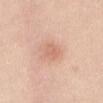Assessment: Part of a total-body skin-imaging series; this lesion was reviewed on a skin check and was not flagged for biopsy. Clinical summary: Measured at roughly 2.5 mm in maximum diameter. This is a white-light tile. A region of skin cropped from a whole-body photographic capture, roughly 15 mm wide. A female patient aged 18 to 22. Automated image analysis of the tile measured border irregularity of about 2.5 on a 0–10 scale, internal color variation of about 1.5 on a 0–10 scale, and radial color variation of about 0.5. Located on the abdomen.